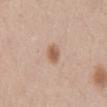{"biopsy_status": "not biopsied; imaged during a skin examination", "patient": {"sex": "female", "age_approx": 35}, "site": "abdomen", "image": {"source": "total-body photography crop", "field_of_view_mm": 15}}